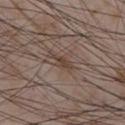notes: no biopsy performed (imaged during a skin exam) | image source: total-body-photography crop, ~15 mm field of view | anatomic site: the front of the torso | subject: male, roughly 75 years of age | tile lighting: white-light illumination | image-analysis metrics: a lesion area of about 3.5 mm², an outline eccentricity of about 0.95 (0 = round, 1 = elongated), and a symmetry-axis asymmetry near 0.3; a lesion color around L≈42 a*≈14 b*≈22 in CIELAB, a lesion–skin lightness drop of about 7, and a normalized border contrast of about 6.5 | diameter: ~4 mm (longest diameter).